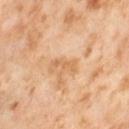The lesion was photographed on a routine skin check and not biopsied; there is no pathology result. A 15 mm crop from a total-body photograph taken for skin-cancer surveillance. Automated image analysis of the tile measured a lesion area of about 3 mm², a shape eccentricity near 0.95, and a symmetry-axis asymmetry near 0.35. The analysis additionally found a mean CIELAB color near L≈66 a*≈22 b*≈40, about 8 CIELAB-L* units darker than the surrounding skin, and a normalized border contrast of about 5.5. It also reported a border-irregularity rating of about 5/10, a color-variation rating of about 0/10, and a peripheral color-asymmetry measure near 0. The patient is a female aged 53–57. Located on the right thigh.